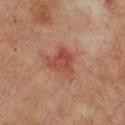  biopsy_status: not biopsied; imaged during a skin examination
  site: chest
  patient:
    sex: male
    age_approx: 70
  image:
    source: total-body photography crop
    field_of_view_mm: 15
  lighting: cross-polarized
  automated_metrics:
    border_irregularity_0_10: 4.0
    color_variation_0_10: 7.0
    peripheral_color_asymmetry: 2.5
    nevus_likeness_0_100: 0
  lesion_size:
    long_diameter_mm_approx: 3.5